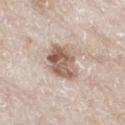Assessment:
Imaged during a routine full-body skin examination; the lesion was not biopsied and no histopathology is available.
Clinical summary:
A male subject, aged approximately 80. Located on the right thigh. A 15 mm crop from a total-body photograph taken for skin-cancer surveillance.